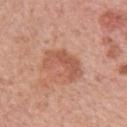Q: Was this lesion biopsied?
A: no biopsy performed (imaged during a skin exam)
Q: Who is the patient?
A: female, roughly 60 years of age
Q: Where on the body is the lesion?
A: the left upper arm
Q: What kind of image is this?
A: ~15 mm tile from a whole-body skin photo
Q: Illumination type?
A: white-light illumination